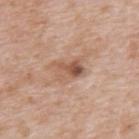Recorded during total-body skin imaging; not selected for excision or biopsy.
The tile uses white-light illumination.
From the upper back.
Longest diameter approximately 3.5 mm.
A male patient aged 48–52.
This image is a 15 mm lesion crop taken from a total-body photograph.